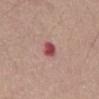Recorded during total-body skin imaging; not selected for excision or biopsy. Cropped from a whole-body photographic skin survey; the tile spans about 15 mm. Located on the mid back. A male subject, aged 68–72.